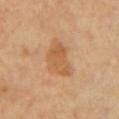Findings:
• diameter: about 5 mm
• lighting: cross-polarized
• automated lesion analysis: a lesion area of about 13 mm² and a symmetry-axis asymmetry near 0.3; border irregularity of about 3 on a 0–10 scale, internal color variation of about 3 on a 0–10 scale, and peripheral color asymmetry of about 1; lesion-presence confidence of about 100/100
• image: ~15 mm tile from a whole-body skin photo
• patient: in their 60s
• anatomic site: the left upper arm